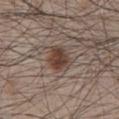Background:
Imaged with white-light lighting. Automated image analysis of the tile measured a lesion area of about 8 mm², an outline eccentricity of about 0.65 (0 = round, 1 = elongated), and a symmetry-axis asymmetry near 0.2. A roughly 15 mm field-of-view crop from a total-body skin photograph. A male patient, roughly 65 years of age. The lesion is located on the chest. About 3.5 mm across.